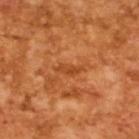Q: Is there a histopathology result?
A: catalogued during a skin exam; not biopsied
Q: What is the imaging modality?
A: 15 mm crop, total-body photography
Q: How was the tile lit?
A: cross-polarized
Q: Automated lesion metrics?
A: a lesion area of about 3 mm², an outline eccentricity of about 0.95 (0 = round, 1 = elongated), and a symmetry-axis asymmetry near 0.4
Q: Patient demographics?
A: male, approximately 65 years of age